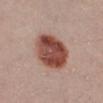Impression:
No biopsy was performed on this lesion — it was imaged during a full skin examination and was not determined to be concerning.
Image and clinical context:
The tile uses white-light illumination. From the right lower leg. A female patient, about 25 years old. Cropped from a total-body skin-imaging series; the visible field is about 15 mm.